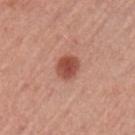Q: Was a biopsy performed?
A: catalogued during a skin exam; not biopsied
Q: How was this image acquired?
A: ~15 mm crop, total-body skin-cancer survey
Q: Automated lesion metrics?
A: border irregularity of about 1.5 on a 0–10 scale and internal color variation of about 4 on a 0–10 scale; a nevus-likeness score of about 100/100 and a detector confidence of about 100 out of 100 that the crop contains a lesion
Q: What lighting was used for the tile?
A: white-light illumination
Q: Patient demographics?
A: male, in their mid- to late 50s
Q: Where on the body is the lesion?
A: the left upper arm
Q: What is the lesion's diameter?
A: about 3 mm